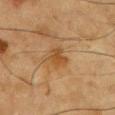A male patient aged 83 or older.
The recorded lesion diameter is about 2.5 mm.
On the chest.
A roughly 15 mm field-of-view crop from a total-body skin photograph.